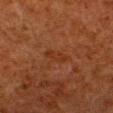Captured during whole-body skin photography for melanoma surveillance; the lesion was not biopsied. The lesion's longest dimension is about 3 mm. From the left lower leg. The tile uses cross-polarized illumination. A male patient aged around 80. A 15 mm close-up extracted from a 3D total-body photography capture.